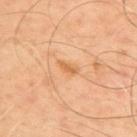Clinical impression: Imaged during a routine full-body skin examination; the lesion was not biopsied and no histopathology is available. Context: The patient is a male aged 48 to 52. The lesion is on the upper back. This image is a 15 mm lesion crop taken from a total-body photograph.